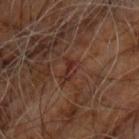notes: no biopsy performed (imaged during a skin exam)
lesion size: ≈2.5 mm
patient: male, approximately 60 years of age
lighting: cross-polarized
imaging modality: ~15 mm crop, total-body skin-cancer survey
body site: the chest